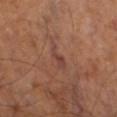The lesion was photographed on a routine skin check and not biopsied; there is no pathology result.
The subject is a male in their mid-60s.
On the left thigh.
Automated image analysis of the tile measured an average lesion color of about L≈39 a*≈21 b*≈24 (CIELAB), roughly 6 lightness units darker than nearby skin, and a lesion-to-skin contrast of about 6 (normalized; higher = more distinct). The analysis additionally found a classifier nevus-likeness of about 15/100.
The lesion's longest dimension is about 3.5 mm.
A roughly 15 mm field-of-view crop from a total-body skin photograph.